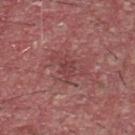subject: male, aged approximately 40 | image: total-body-photography crop, ~15 mm field of view | site: the front of the torso.